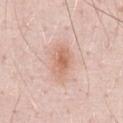Clinical summary:
On the abdomen. A male patient about 50 years old. A close-up tile cropped from a whole-body skin photograph, about 15 mm across.